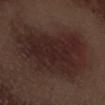Case summary:
– workup — no biopsy performed (imaged during a skin exam)
– patient — male, in their 70s
– tile lighting — white-light illumination
– size — about 11.5 mm
– body site — the left forearm
– automated lesion analysis — border irregularity of about 4 on a 0–10 scale, internal color variation of about 4 on a 0–10 scale, and peripheral color asymmetry of about 1.5; a detector confidence of about 100 out of 100 that the crop contains a lesion
– image — total-body-photography crop, ~15 mm field of view